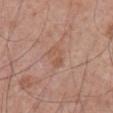patient — male, aged 68–72
acquisition — 15 mm crop, total-body photography
tile lighting — white-light
TBP lesion metrics — a footprint of about 4 mm², an eccentricity of roughly 0.9, and two-axis asymmetry of about 0.4; peripheral color asymmetry of about 1
lesion diameter — ≈3 mm
location — the abdomen